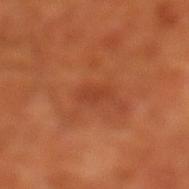A 15 mm close-up tile from a total-body photography series done for melanoma screening.
About 2.5 mm across.
A male subject, aged around 70.
The lesion-visualizer software estimated roughly 6 lightness units darker than nearby skin and a normalized border contrast of about 4.5.
The lesion is on the left lower leg.